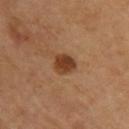notes = total-body-photography surveillance lesion; no biopsy | tile lighting = cross-polarized | lesion diameter = ~2.5 mm (longest diameter) | image-analysis metrics = a lesion area of about 5.5 mm² and two-axis asymmetry of about 0.2; a lesion color around L≈41 a*≈23 b*≈34 in CIELAB, about 13 CIELAB-L* units darker than the surrounding skin, and a normalized border contrast of about 10; a classifier nevus-likeness of about 100/100 and a detector confidence of about 100 out of 100 that the crop contains a lesion | site = the upper back | acquisition = total-body-photography crop, ~15 mm field of view.